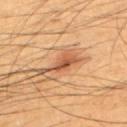notes — imaged on a skin check; not biopsied | illumination — cross-polarized illumination | imaging modality — ~15 mm crop, total-body skin-cancer survey | patient — male, aged approximately 50 | diameter — about 5 mm | automated metrics — a mean CIELAB color near L≈54 a*≈23 b*≈36, roughly 11 lightness units darker than nearby skin, and a lesion-to-skin contrast of about 8 (normalized; higher = more distinct); a nevus-likeness score of about 95/100 and a detector confidence of about 100 out of 100 that the crop contains a lesion | location — the upper back.